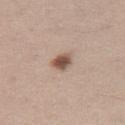Findings:
- workup: catalogued during a skin exam; not biopsied